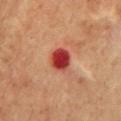Impression:
Captured during whole-body skin photography for melanoma surveillance; the lesion was not biopsied.
Clinical summary:
From the chest. The subject is a male aged 58 to 62. Longest diameter approximately 3 mm. Automated image analysis of the tile measured an area of roughly 6.5 mm², a shape eccentricity near 0.5, and a symmetry-axis asymmetry near 0.2. The software also gave a classifier nevus-likeness of about 0/100 and a lesion-detection confidence of about 100/100. Captured under cross-polarized illumination. This image is a 15 mm lesion crop taken from a total-body photograph.